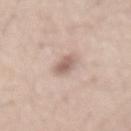biopsy_status: not biopsied; imaged during a skin examination
automated_metrics:
  area_mm2_approx: 4.5
  eccentricity: 0.75
  cielab_L: 61
  cielab_a: 16
  cielab_b: 24
  vs_skin_darker_L: 12.0
  nevus_likeness_0_100: 30
  lesion_detection_confidence_0_100: 100
site: lower back
lesion_size:
  long_diameter_mm_approx: 3.0
image:
  source: total-body photography crop
  field_of_view_mm: 15
patient:
  sex: male
  age_approx: 40